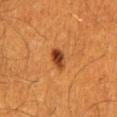Assessment: This lesion was catalogued during total-body skin photography and was not selected for biopsy. Background: A male subject roughly 60 years of age. Imaged with cross-polarized lighting. On the lower back. Cropped from a total-body skin-imaging series; the visible field is about 15 mm. Automated tile analysis of the lesion measured a nevus-likeness score of about 95/100 and lesion-presence confidence of about 100/100.